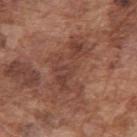Recorded during total-body skin imaging; not selected for excision or biopsy.
From the arm.
About 8 mm across.
A close-up tile cropped from a whole-body skin photograph, about 15 mm across.
The patient is a male roughly 75 years of age.
The lesion-visualizer software estimated a footprint of about 20 mm², a shape eccentricity near 0.85, and two-axis asymmetry of about 0.5. And it measured a lesion–skin lightness drop of about 8 and a normalized lesion–skin contrast near 6.5. The analysis additionally found border irregularity of about 8.5 on a 0–10 scale, a color-variation rating of about 4.5/10, and radial color variation of about 1.5.
The tile uses white-light illumination.